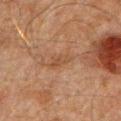workup: no biopsy performed (imaged during a skin exam)
patient: male, roughly 60 years of age
diameter: ≈3.5 mm
imaging modality: total-body-photography crop, ~15 mm field of view
lighting: cross-polarized illumination
anatomic site: the chest